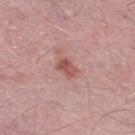workup: no biopsy performed (imaged during a skin exam) | patient: male, roughly 50 years of age | automated metrics: a lesion area of about 4 mm², an eccentricity of roughly 0.8, and two-axis asymmetry of about 0.25; border irregularity of about 2.5 on a 0–10 scale, internal color variation of about 3.5 on a 0–10 scale, and peripheral color asymmetry of about 1.5 | anatomic site: the left thigh | illumination: white-light | diameter: ~2.5 mm (longest diameter) | image source: ~15 mm tile from a whole-body skin photo.